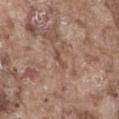  biopsy_status: not biopsied; imaged during a skin examination
  lesion_size:
    long_diameter_mm_approx: 2.5
  lighting: white-light
  site: abdomen
  patient:
    sex: male
    age_approx: 75
  image:
    source: total-body photography crop
    field_of_view_mm: 15